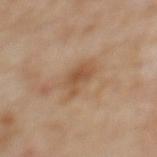Assessment:
Recorded during total-body skin imaging; not selected for excision or biopsy.
Image and clinical context:
The tile uses cross-polarized illumination. Cropped from a total-body skin-imaging series; the visible field is about 15 mm. From the upper back. The total-body-photography lesion software estimated a footprint of about 7 mm², an outline eccentricity of about 0.75 (0 = round, 1 = elongated), and a symmetry-axis asymmetry near 0.25. And it measured a border-irregularity rating of about 3/10, a color-variation rating of about 2.5/10, and peripheral color asymmetry of about 0.5. The software also gave an automated nevus-likeness rating near 0 out of 100 and lesion-presence confidence of about 100/100. A female subject aged 58 to 62. Approximately 3.5 mm at its widest.